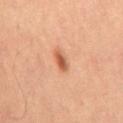Clinical impression: Captured during whole-body skin photography for melanoma surveillance; the lesion was not biopsied. Clinical summary: The subject is a male in their 60s. Approximately 2.5 mm at its widest. Cropped from a total-body skin-imaging series; the visible field is about 15 mm. Imaged with cross-polarized lighting. On the abdomen.